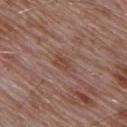biopsy_status: not biopsied; imaged during a skin examination
lesion_size:
  long_diameter_mm_approx: 2.5
site: upper back
image:
  source: total-body photography crop
  field_of_view_mm: 15
automated_metrics:
  area_mm2_approx: 3.0
  eccentricity: 0.8
  shape_asymmetry: 0.35
  border_irregularity_0_10: 3.5
  peripheral_color_asymmetry: 0.5
  nevus_likeness_0_100: 5
  lesion_detection_confidence_0_100: 100
patient:
  sex: male
  age_approx: 55